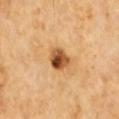notes = total-body-photography surveillance lesion; no biopsy | subject = male, approximately 60 years of age | automated lesion analysis = a border-irregularity rating of about 2/10, internal color variation of about 9 on a 0–10 scale, and peripheral color asymmetry of about 3 | lesion diameter = ~3 mm (longest diameter) | image = total-body-photography crop, ~15 mm field of view | site = the upper back | lighting = cross-polarized illumination.